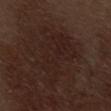notes — imaged on a skin check; not biopsied | subject — male, in their 70s | body site — the abdomen | automated lesion analysis — a lesion area of about 25 mm² and an outline eccentricity of about 0.8 (0 = round, 1 = elongated); a lesion color around L≈21 a*≈16 b*≈19 in CIELAB and a lesion–skin lightness drop of about 4; a classifier nevus-likeness of about 10/100 and a detector confidence of about 100 out of 100 that the crop contains a lesion | acquisition — ~15 mm tile from a whole-body skin photo.